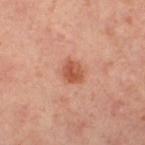This lesion was catalogued during total-body skin photography and was not selected for biopsy. The tile uses cross-polarized illumination. A female subject, roughly 40 years of age. From the left lower leg. An algorithmic analysis of the crop reported an outline eccentricity of about 0.55 (0 = round, 1 = elongated) and a shape-asymmetry score of about 0.25 (0 = symmetric). It also reported a mean CIELAB color near L≈54 a*≈28 b*≈34, roughly 11 lightness units darker than nearby skin, and a lesion-to-skin contrast of about 8 (normalized; higher = more distinct). It also reported a border-irregularity rating of about 2/10, a within-lesion color-variation index near 3.5/10, and a peripheral color-asymmetry measure near 1. And it measured an automated nevus-likeness rating near 95 out of 100 and a lesion-detection confidence of about 100/100. A 15 mm close-up tile from a total-body photography series done for melanoma screening.